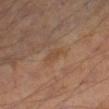workup = total-body-photography surveillance lesion; no biopsy | size = ~2.5 mm (longest diameter) | patient = male, aged 53–57 | automated lesion analysis = an automated nevus-likeness rating near 0 out of 100 and a lesion-detection confidence of about 100/100 | image = total-body-photography crop, ~15 mm field of view | tile lighting = cross-polarized | location = the right thigh.